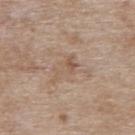Q: Was this lesion biopsied?
A: total-body-photography surveillance lesion; no biopsy
Q: Where on the body is the lesion?
A: the upper back
Q: Automated lesion metrics?
A: a shape eccentricity near 0.85; an average lesion color of about L≈55 a*≈16 b*≈28 (CIELAB), roughly 6 lightness units darker than nearby skin, and a lesion-to-skin contrast of about 4.5 (normalized; higher = more distinct); border irregularity of about 6.5 on a 0–10 scale, a color-variation rating of about 3/10, and peripheral color asymmetry of about 1
Q: Patient demographics?
A: female, in their mid-70s
Q: Lesion size?
A: about 3.5 mm
Q: Illumination type?
A: white-light
Q: How was this image acquired?
A: ~15 mm crop, total-body skin-cancer survey